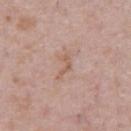Notes:
• notes — no biopsy performed (imaged during a skin exam)
• image source — ~15 mm tile from a whole-body skin photo
• diameter — ≈3 mm
• lighting — white-light illumination
• anatomic site — the chest
• subject — male, aged 38 to 42